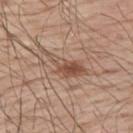Clinical impression:
The lesion was tiled from a total-body skin photograph and was not biopsied.
Context:
A close-up tile cropped from a whole-body skin photograph, about 15 mm across. A male subject in their mid- to late 60s. The lesion is on the upper back.